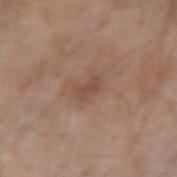lesion_size:
  long_diameter_mm_approx: 2.5
automated_metrics:
  eccentricity: 0.8
  shape_asymmetry: 0.3
  vs_skin_contrast_norm: 5.5
  border_irregularity_0_10: 3.5
  peripheral_color_asymmetry: 0.5
patient:
  sex: male
  age_approx: 80
image:
  source: total-body photography crop
  field_of_view_mm: 15
site: right forearm